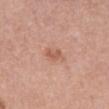biopsy status = total-body-photography surveillance lesion; no biopsy
image = ~15 mm crop, total-body skin-cancer survey
site = the back
lesion diameter = about 2.5 mm
illumination = white-light illumination
subject = male, in their 70s